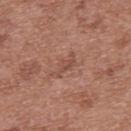Image and clinical context: The lesion is on the back. A 15 mm crop from a total-body photograph taken for skin-cancer surveillance. Automated image analysis of the tile measured a nevus-likeness score of about 0/100 and a detector confidence of about 100 out of 100 that the crop contains a lesion. Imaged with white-light lighting. A female subject, in their 40s. Longest diameter approximately 3.5 mm.